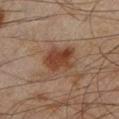Context:
The lesion is on the left lower leg. An algorithmic analysis of the crop reported internal color variation of about 3.5 on a 0–10 scale and a peripheral color-asymmetry measure near 1. And it measured a nevus-likeness score of about 75/100. Cropped from a total-body skin-imaging series; the visible field is about 15 mm. The patient is a male approximately 45 years of age.